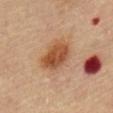Recorded during total-body skin imaging; not selected for excision or biopsy. Measured at roughly 5 mm in maximum diameter. The lesion is located on the chest. A male patient, in their mid- to late 80s or older. Automated image analysis of the tile measured a footprint of about 12 mm², an eccentricity of roughly 0.75, and a symmetry-axis asymmetry near 0.15. The analysis additionally found a mean CIELAB color near L≈49 a*≈23 b*≈35, roughly 12 lightness units darker than nearby skin, and a normalized lesion–skin contrast near 9. The software also gave border irregularity of about 2 on a 0–10 scale, internal color variation of about 4.5 on a 0–10 scale, and radial color variation of about 1.5. It also reported lesion-presence confidence of about 100/100. A roughly 15 mm field-of-view crop from a total-body skin photograph.